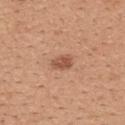Notes:
* follow-up: imaged on a skin check; not biopsied
* automated metrics: a footprint of about 4.5 mm², a shape eccentricity near 0.75, and a shape-asymmetry score of about 0.25 (0 = symmetric); a mean CIELAB color near L≈54 a*≈23 b*≈32, a lesion–skin lightness drop of about 11, and a normalized lesion–skin contrast near 7.5; a border-irregularity rating of about 2/10, a color-variation rating of about 2/10, and radial color variation of about 0.5; a classifier nevus-likeness of about 80/100 and a detector confidence of about 100 out of 100 that the crop contains a lesion
* subject: female, aged around 30
* image: ~15 mm tile from a whole-body skin photo
* location: the back
* lighting: white-light
* diameter: about 3 mm